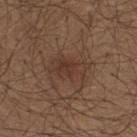The lesion was tiled from a total-body skin photograph and was not biopsied.
The patient is a male aged around 25.
From the upper back.
A 15 mm close-up tile from a total-body photography series done for melanoma screening.